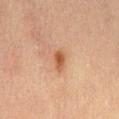biopsy status=no biopsy performed (imaged during a skin exam); patient=female, in their 60s; image=~15 mm tile from a whole-body skin photo; body site=the abdomen; illumination=cross-polarized illumination.